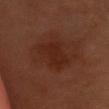Q: Was this lesion biopsied?
A: total-body-photography surveillance lesion; no biopsy
Q: What did automated image analysis measure?
A: an average lesion color of about L≈19 a*≈19 b*≈22 (CIELAB) and a lesion–skin lightness drop of about 4; a nevus-likeness score of about 0/100
Q: What is the imaging modality?
A: 15 mm crop, total-body photography
Q: Lesion size?
A: ~4.5 mm (longest diameter)
Q: Patient demographics?
A: male, aged approximately 55
Q: What is the anatomic site?
A: the head or neck
Q: How was the tile lit?
A: cross-polarized illumination